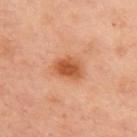Q: Is there a histopathology result?
A: catalogued during a skin exam; not biopsied
Q: Where on the body is the lesion?
A: the chest
Q: Illumination type?
A: cross-polarized illumination
Q: Patient demographics?
A: female, approximately 55 years of age
Q: How was this image acquired?
A: total-body-photography crop, ~15 mm field of view
Q: How large is the lesion?
A: ~3.5 mm (longest diameter)